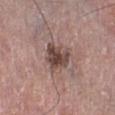Q: Is there a histopathology result?
A: no biopsy performed (imaged during a skin exam)
Q: What are the patient's age and sex?
A: male, aged approximately 75
Q: Lesion location?
A: the left lower leg
Q: How was this image acquired?
A: 15 mm crop, total-body photography